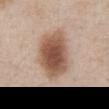This lesion was catalogued during total-body skin photography and was not selected for biopsy.
Captured under white-light illumination.
A 15 mm crop from a total-body photograph taken for skin-cancer surveillance.
The lesion is on the abdomen.
A male subject, aged 58 to 62.
Automated tile analysis of the lesion measured a lesion area of about 23 mm² and a shape-asymmetry score of about 0.2 (0 = symmetric). It also reported an average lesion color of about L≈54 a*≈19 b*≈29 (CIELAB), a lesion–skin lightness drop of about 16, and a lesion-to-skin contrast of about 10.5 (normalized; higher = more distinct). It also reported border irregularity of about 2 on a 0–10 scale, a within-lesion color-variation index near 6/10, and radial color variation of about 1.5. The analysis additionally found an automated nevus-likeness rating near 100 out of 100 and a lesion-detection confidence of about 100/100.